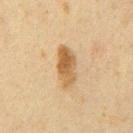This lesion was catalogued during total-body skin photography and was not selected for biopsy.
The subject is a male aged approximately 60.
A lesion tile, about 15 mm wide, cut from a 3D total-body photograph.
The lesion is on the chest.